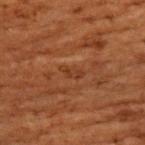Q: Was a biopsy performed?
A: no biopsy performed (imaged during a skin exam)
Q: What is the lesion's diameter?
A: ~2.5 mm (longest diameter)
Q: How was this image acquired?
A: ~15 mm tile from a whole-body skin photo
Q: How was the tile lit?
A: cross-polarized illumination
Q: Who is the patient?
A: female, aged approximately 80
Q: Where on the body is the lesion?
A: the chest